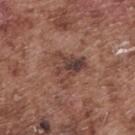  biopsy_status: not biopsied; imaged during a skin examination
  patient:
    sex: male
    age_approx: 75
  lighting: white-light
  lesion_size:
    long_diameter_mm_approx: 4.5
  image:
    source: total-body photography crop
    field_of_view_mm: 15
  site: upper back